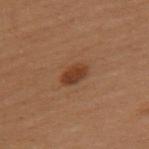<case>
<biopsy_status>not biopsied; imaged during a skin examination</biopsy_status>
<patient>
  <sex>female</sex>
  <age_approx>50</age_approx>
</patient>
<lesion_size>
  <long_diameter_mm_approx>3.0</long_diameter_mm_approx>
</lesion_size>
<site>left upper arm</site>
<image>
  <source>total-body photography crop</source>
  <field_of_view_mm>15</field_of_view_mm>
</image>
<lighting>cross-polarized</lighting>
</case>